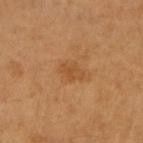Impression:
Recorded during total-body skin imaging; not selected for excision or biopsy.
Clinical summary:
Cropped from a total-body skin-imaging series; the visible field is about 15 mm. From the left arm. The patient is a female approximately 45 years of age.